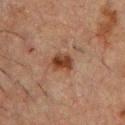Impression:
Imaged during a routine full-body skin examination; the lesion was not biopsied and no histopathology is available.
Clinical summary:
An algorithmic analysis of the crop reported an area of roughly 5 mm², a shape eccentricity near 0.65, and two-axis asymmetry of about 0.2. The software also gave a classifier nevus-likeness of about 95/100 and a detector confidence of about 100 out of 100 that the crop contains a lesion. Longest diameter approximately 2.5 mm. From the chest. Cropped from a total-body skin-imaging series; the visible field is about 15 mm. Imaged with cross-polarized lighting. The patient is a male aged 48 to 52.